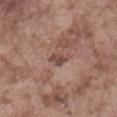biopsy status = total-body-photography surveillance lesion; no biopsy
lighting = white-light
body site = the front of the torso
acquisition = total-body-photography crop, ~15 mm field of view
patient = male, aged around 75